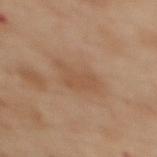Part of a total-body skin-imaging series; this lesion was reviewed on a skin check and was not flagged for biopsy. Captured under cross-polarized illumination. A female subject about 55 years old. Cropped from a whole-body photographic skin survey; the tile spans about 15 mm. On the upper back.